A male subject, about 85 years old or older. Approximately 2 mm at its widest. A roughly 15 mm field-of-view crop from a total-body skin photograph. The lesion is on the mid back. Imaged with white-light lighting. Automated image analysis of the tile measured a symmetry-axis asymmetry near 0.4. It also reported a border-irregularity index near 4/10, a color-variation rating of about 0/10, and a peripheral color-asymmetry measure near 0. It also reported a nevus-likeness score of about 0/100 and a lesion-detection confidence of about 0/100 — 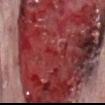On excision, pathology confirmed a malignant lesion: nodular basal cell carcinoma.